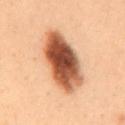Approximately 8.5 mm at its widest. From the back. A female patient, approximately 50 years of age. A lesion tile, about 15 mm wide, cut from a 3D total-body photograph. The total-body-photography lesion software estimated an automated nevus-likeness rating near 100 out of 100 and a detector confidence of about 100 out of 100 that the crop contains a lesion. Imaged with cross-polarized lighting.